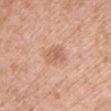patient:
  sex: female
  age_approx: 60
lesion_size:
  long_diameter_mm_approx: 3.5
site: front of the torso
image:
  source: total-body photography crop
  field_of_view_mm: 15